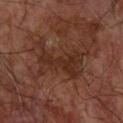No biopsy was performed on this lesion — it was imaged during a full skin examination and was not determined to be concerning.
The lesion is on the right forearm.
This image is a 15 mm lesion crop taken from a total-body photograph.
Imaged with cross-polarized lighting.
Approximately 6.5 mm at its widest.
A male subject aged around 65.